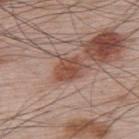Part of a total-body skin-imaging series; this lesion was reviewed on a skin check and was not flagged for biopsy. Automated tile analysis of the lesion measured an area of roughly 8 mm², an outline eccentricity of about 0.7 (0 = round, 1 = elongated), and a symmetry-axis asymmetry near 0.15. The analysis additionally found a lesion-detection confidence of about 100/100. A male subject, in their mid-60s. The lesion's longest dimension is about 3.5 mm. Cropped from a total-body skin-imaging series; the visible field is about 15 mm. The lesion is on the upper back. Imaged with white-light lighting.